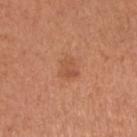Captured during whole-body skin photography for melanoma surveillance; the lesion was not biopsied.